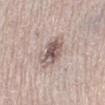The lesion was photographed on a routine skin check and not biopsied; there is no pathology result.
A 15 mm close-up extracted from a 3D total-body photography capture.
Captured under white-light illumination.
About 4 mm across.
A male subject in their mid-70s.
From the lower back.